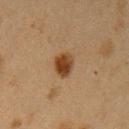acquisition — 15 mm crop, total-body photography; subject — female, in their 40s; body site — the left upper arm; lighting — cross-polarized illumination; image-analysis metrics — a border-irregularity index near 2/10, a color-variation rating of about 4/10, and radial color variation of about 1.